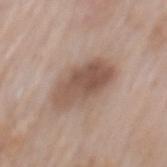follow-up = no biopsy performed (imaged during a skin exam) | acquisition = 15 mm crop, total-body photography | patient = male, aged 58–62 | size = ~6 mm (longest diameter) | tile lighting = white-light | automated metrics = a lesion color around L≈53 a*≈17 b*≈25 in CIELAB and a lesion–skin lightness drop of about 12; a classifier nevus-likeness of about 20/100 and a lesion-detection confidence of about 100/100 | site = the mid back.